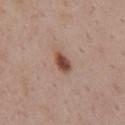<tbp_lesion>
  <lighting>white-light</lighting>
  <lesion_size>
    <long_diameter_mm_approx>3.0</long_diameter_mm_approx>
  </lesion_size>
  <automated_metrics>
    <area_mm2_approx>4.5</area_mm2_approx>
    <eccentricity>0.8</eccentricity>
    <shape_asymmetry>0.15</shape_asymmetry>
    <cielab_L>49</cielab_L>
    <cielab_a>20</cielab_a>
    <cielab_b>27</cielab_b>
    <vs_skin_darker_L>13.0</vs_skin_darker_L>
    <nevus_likeness_0_100>100</nevus_likeness_0_100>
    <lesion_detection_confidence_0_100>100</lesion_detection_confidence_0_100>
  </automated_metrics>
  <site>chest</site>
  <image>
    <source>total-body photography crop</source>
    <field_of_view_mm>15</field_of_view_mm>
  </image>
  <patient>
    <sex>female</sex>
    <age_approx>55</age_approx>
  </patient>
</tbp_lesion>